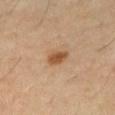No biopsy was performed on this lesion — it was imaged during a full skin examination and was not determined to be concerning.
This is a cross-polarized tile.
The subject is a male aged 58–62.
A 15 mm close-up tile from a total-body photography series done for melanoma screening.
Approximately 3 mm at its widest.
From the right thigh.